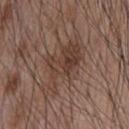Impression: Imaged during a routine full-body skin examination; the lesion was not biopsied and no histopathology is available. Clinical summary: Measured at roughly 8.5 mm in maximum diameter. This is a white-light tile. The lesion is located on the front of the torso. A 15 mm close-up tile from a total-body photography series done for melanoma screening. A male patient, aged 53 to 57.